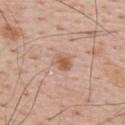| key | value |
|---|---|
| workup | total-body-photography surveillance lesion; no biopsy |
| site | the upper back |
| acquisition | 15 mm crop, total-body photography |
| illumination | white-light illumination |
| lesion size | about 2.5 mm |
| automated lesion analysis | a lesion color around L≈59 a*≈19 b*≈30 in CIELAB, about 10 CIELAB-L* units darker than the surrounding skin, and a lesion-to-skin contrast of about 7 (normalized; higher = more distinct); a border-irregularity index near 2/10 and internal color variation of about 3.5 on a 0–10 scale |
| subject | male, in their mid- to late 60s |